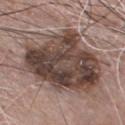notes=total-body-photography surveillance lesion; no biopsy
site=the chest
image source=~15 mm tile from a whole-body skin photo
patient=male, approximately 75 years of age
illumination=white-light
lesion diameter=~11.5 mm (longest diameter)